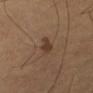| key | value |
|---|---|
| follow-up | total-body-photography surveillance lesion; no biopsy |
| imaging modality | total-body-photography crop, ~15 mm field of view |
| lesion diameter | about 2.5 mm |
| patient | male, aged around 65 |
| tile lighting | cross-polarized |
| location | the arm |
| image-analysis metrics | an area of roughly 4 mm² and an outline eccentricity of about 0.7 (0 = round, 1 = elongated); a lesion color around L≈34 a*≈17 b*≈25 in CIELAB and a normalized border contrast of about 8 |